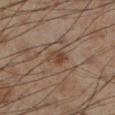This lesion was catalogued during total-body skin photography and was not selected for biopsy. The lesion is located on the left lower leg. The tile uses cross-polarized illumination. The lesion's longest dimension is about 3 mm. Cropped from a total-body skin-imaging series; the visible field is about 15 mm. The subject is a male aged approximately 55.